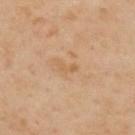Q: Was this lesion biopsied?
A: catalogued during a skin exam; not biopsied
Q: What is the anatomic site?
A: the upper back
Q: What is the imaging modality?
A: total-body-photography crop, ~15 mm field of view
Q: What are the patient's age and sex?
A: male, in their mid- to late 50s
Q: What is the lesion's diameter?
A: ≈2.5 mm